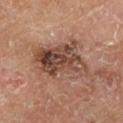Q: Was a biopsy performed?
A: no biopsy performed (imaged during a skin exam)
Q: Illumination type?
A: cross-polarized
Q: Automated lesion metrics?
A: an area of roughly 24 mm² and a symmetry-axis asymmetry near 0.3; a lesion color around L≈46 a*≈20 b*≈27 in CIELAB, about 12 CIELAB-L* units darker than the surrounding skin, and a normalized lesion–skin contrast near 9; a border-irregularity index near 4/10; lesion-presence confidence of about 100/100
Q: What is the lesion's diameter?
A: ≈7 mm
Q: What kind of image is this?
A: 15 mm crop, total-body photography
Q: Who is the patient?
A: male, aged 63 to 67
Q: Where on the body is the lesion?
A: the right lower leg